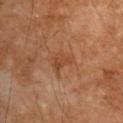Q: Was this lesion biopsied?
A: no biopsy performed (imaged during a skin exam)
Q: Patient demographics?
A: male, aged approximately 55
Q: What lighting was used for the tile?
A: cross-polarized illumination
Q: What kind of image is this?
A: total-body-photography crop, ~15 mm field of view
Q: Where on the body is the lesion?
A: the arm